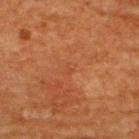<lesion>
  <biopsy_status>not biopsied; imaged during a skin examination</biopsy_status>
</lesion>